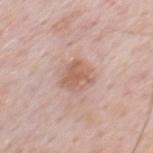- follow-up · imaged on a skin check; not biopsied
- TBP lesion metrics · a lesion-detection confidence of about 100/100
- image source · 15 mm crop, total-body photography
- subject · male, aged around 80
- site · the chest